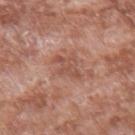Clinical impression:
The lesion was photographed on a routine skin check and not biopsied; there is no pathology result.
Clinical summary:
The patient is a male aged 58–62. The lesion is on the left forearm. A close-up tile cropped from a whole-body skin photograph, about 15 mm across.